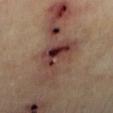<tbp_lesion>
  <biopsy_status>not biopsied; imaged during a skin examination</biopsy_status>
  <patient>
    <age_approx>60</age_approx>
  </patient>
  <image>
    <source>total-body photography crop</source>
    <field_of_view_mm>15</field_of_view_mm>
  </image>
  <lighting>cross-polarized</lighting>
  <lesion_size>
    <long_diameter_mm_approx>6.0</long_diameter_mm_approx>
  </lesion_size>
  <site>left lower leg</site>
</tbp_lesion>